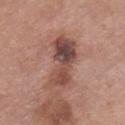Assessment:
The lesion was photographed on a routine skin check and not biopsied; there is no pathology result.
Context:
A male patient, roughly 75 years of age. This image is a 15 mm lesion crop taken from a total-body photograph. The lesion is on the chest.